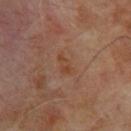  biopsy_status: not biopsied; imaged during a skin examination
  image:
    source: total-body photography crop
    field_of_view_mm: 15
  site: upper back
  patient:
    sex: male
    age_approx: 65
  lesion_size:
    long_diameter_mm_approx: 3.0
  automated_metrics:
    cielab_L: 41
    cielab_a: 20
    cielab_b: 29
    vs_skin_darker_L: 5.0
    vs_skin_contrast_norm: 5.5
    border_irregularity_0_10: 3.5
    color_variation_0_10: 4.0
    peripheral_color_asymmetry: 1.5
    nevus_likeness_0_100: 0
  lighting: cross-polarized